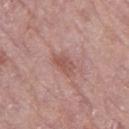{
  "lighting": "white-light",
  "site": "leg",
  "lesion_size": {
    "long_diameter_mm_approx": 3.0
  },
  "image": {
    "source": "total-body photography crop",
    "field_of_view_mm": 15
  },
  "patient": {
    "sex": "female",
    "age_approx": 75
  }
}